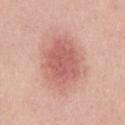Captured during whole-body skin photography for melanoma surveillance; the lesion was not biopsied.
The lesion is on the chest.
The recorded lesion diameter is about 6.5 mm.
The total-body-photography lesion software estimated a lesion area of about 23 mm², an outline eccentricity of about 0.7 (0 = round, 1 = elongated), and a shape-asymmetry score of about 0.15 (0 = symmetric). The software also gave a lesion color around L≈60 a*≈27 b*≈26 in CIELAB, about 11 CIELAB-L* units darker than the surrounding skin, and a normalized lesion–skin contrast near 7. And it measured a border-irregularity rating of about 2/10 and radial color variation of about 1.
This image is a 15 mm lesion crop taken from a total-body photograph.
A male patient, aged 53–57.